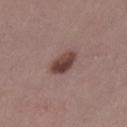size: about 3.5 mm
subject: female, aged around 45
image source: ~15 mm crop, total-body skin-cancer survey
automated metrics: a lesion color around L≈42 a*≈19 b*≈21 in CIELAB, about 13 CIELAB-L* units darker than the surrounding skin, and a lesion-to-skin contrast of about 10 (normalized; higher = more distinct)
anatomic site: the left thigh